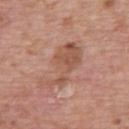Assessment:
The lesion was tiled from a total-body skin photograph and was not biopsied.
Clinical summary:
A male patient about 70 years old. On the upper back. The lesion's longest dimension is about 7 mm. Cropped from a total-body skin-imaging series; the visible field is about 15 mm.